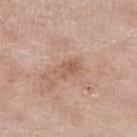The lesion was tiled from a total-body skin photograph and was not biopsied. The lesion's longest dimension is about 3 mm. A female patient, roughly 75 years of age. From the right lower leg. The lesion-visualizer software estimated an area of roughly 4.5 mm², an eccentricity of roughly 0.8, and a symmetry-axis asymmetry near 0.3. The software also gave a lesion color around L≈57 a*≈20 b*≈30 in CIELAB and a normalized border contrast of about 6. The software also gave a border-irregularity index near 3/10 and a peripheral color-asymmetry measure near 1. A 15 mm close-up tile from a total-body photography series done for melanoma screening. This is a white-light tile.